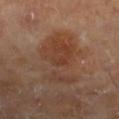follow-up: total-body-photography surveillance lesion; no biopsy | body site: the left lower leg | image source: ~15 mm crop, total-body skin-cancer survey | subject: male, in their mid-60s.